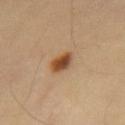Impression: Captured during whole-body skin photography for melanoma surveillance; the lesion was not biopsied. Background: Automated image analysis of the tile measured a footprint of about 5 mm², an outline eccentricity of about 0.55 (0 = round, 1 = elongated), and two-axis asymmetry of about 0.25. And it measured a within-lesion color-variation index near 5/10 and radial color variation of about 1.5. The tile uses cross-polarized illumination. The subject is a male aged approximately 55. On the mid back. A roughly 15 mm field-of-view crop from a total-body skin photograph.